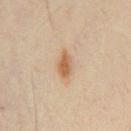Part of a total-body skin-imaging series; this lesion was reviewed on a skin check and was not flagged for biopsy. A close-up tile cropped from a whole-body skin photograph, about 15 mm across. The subject is a male about 50 years old. Located on the chest.